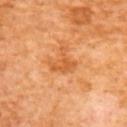This lesion was catalogued during total-body skin photography and was not selected for biopsy. About 3 mm across. Automated image analysis of the tile measured a footprint of about 6 mm² and two-axis asymmetry of about 0.5. And it measured roughly 8 lightness units darker than nearby skin and a normalized border contrast of about 6. The analysis additionally found a border-irregularity rating of about 7/10, a color-variation rating of about 2/10, and a peripheral color-asymmetry measure near 0.5. A female subject, aged around 65. Captured under cross-polarized illumination. Located on the upper back. A close-up tile cropped from a whole-body skin photograph, about 15 mm across.